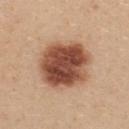No biopsy was performed on this lesion — it was imaged during a full skin examination and was not determined to be concerning.
Cropped from a total-body skin-imaging series; the visible field is about 15 mm.
The lesion is located on the upper back.
A female patient, in their mid-20s.
The total-body-photography lesion software estimated a border-irregularity index near 1.5/10, a color-variation rating of about 7/10, and a peripheral color-asymmetry measure near 2.
Captured under white-light illumination.
The recorded lesion diameter is about 6 mm.